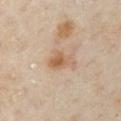Assessment: Imaged during a routine full-body skin examination; the lesion was not biopsied and no histopathology is available. Background: The lesion is on the chest. The total-body-photography lesion software estimated a lesion area of about 6.5 mm² and a symmetry-axis asymmetry near 0.45. The analysis additionally found an automated nevus-likeness rating near 5 out of 100 and a detector confidence of about 100 out of 100 that the crop contains a lesion. A 15 mm close-up extracted from a 3D total-body photography capture. The lesion's longest dimension is about 3.5 mm. A female patient, about 60 years old.